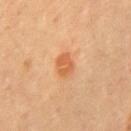This lesion was catalogued during total-body skin photography and was not selected for biopsy. This is a cross-polarized tile. On the front of the torso. About 2.5 mm across. A female patient, approximately 55 years of age. A 15 mm close-up tile from a total-body photography series done for melanoma screening.